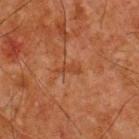The lesion was tiled from a total-body skin photograph and was not biopsied. The lesion is located on the upper back. The lesion's longest dimension is about 2.5 mm. A 15 mm close-up tile from a total-body photography series done for melanoma screening. A male patient, roughly 65 years of age. This is a cross-polarized tile. The lesion-visualizer software estimated an area of roughly 2 mm², an outline eccentricity of about 0.95 (0 = round, 1 = elongated), and a symmetry-axis asymmetry near 0.55.